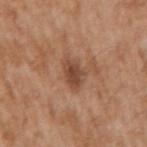workup: imaged on a skin check; not biopsied | patient: male, aged approximately 65 | location: the arm | lighting: white-light illumination | image source: 15 mm crop, total-body photography | automated lesion analysis: an area of roughly 6 mm² and a shape eccentricity near 0.8.